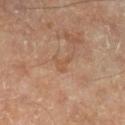Notes:
– notes — no biopsy performed (imaged during a skin exam)
– body site — the right lower leg
– patient — about 55 years old
– image — ~15 mm crop, total-body skin-cancer survey
– illumination — cross-polarized